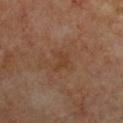workup = catalogued during a skin exam; not biopsied | acquisition = ~15 mm crop, total-body skin-cancer survey | location = the chest | illumination = cross-polarized | size = ~2.5 mm (longest diameter) | patient = female, approximately 60 years of age.